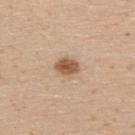notes=total-body-photography surveillance lesion; no biopsy
site=the upper back
diameter=~3 mm (longest diameter)
automated metrics=a lesion area of about 5.5 mm² and an eccentricity of roughly 0.6; a lesion color around L≈56 a*≈19 b*≈33 in CIELAB, about 13 CIELAB-L* units darker than the surrounding skin, and a normalized border contrast of about 9; a peripheral color-asymmetry measure near 1; a detector confidence of about 100 out of 100 that the crop contains a lesion
tile lighting=white-light
patient=male, roughly 30 years of age
acquisition=~15 mm tile from a whole-body skin photo